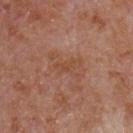Impression: Recorded during total-body skin imaging; not selected for excision or biopsy. Acquisition and patient details: The lesion's longest dimension is about 3.5 mm. The subject is a male about 65 years old. The tile uses white-light illumination. The lesion is on the front of the torso. A 15 mm crop from a total-body photograph taken for skin-cancer surveillance.